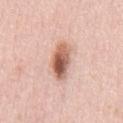Captured during whole-body skin photography for melanoma surveillance; the lesion was not biopsied. A lesion tile, about 15 mm wide, cut from a 3D total-body photograph. The lesion is located on the chest. The lesion's longest dimension is about 5 mm. The patient is a male aged 38 to 42. The lesion-visualizer software estimated about 17 CIELAB-L* units darker than the surrounding skin. The analysis additionally found a classifier nevus-likeness of about 100/100 and a detector confidence of about 100 out of 100 that the crop contains a lesion. The tile uses white-light illumination.